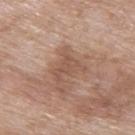This lesion was catalogued during total-body skin photography and was not selected for biopsy. From the upper back. Automated tile analysis of the lesion measured a lesion color around L≈53 a*≈19 b*≈28 in CIELAB, roughly 7 lightness units darker than nearby skin, and a normalized border contrast of about 5. And it measured border irregularity of about 6.5 on a 0–10 scale, internal color variation of about 1.5 on a 0–10 scale, and peripheral color asymmetry of about 0.5. And it measured a classifier nevus-likeness of about 0/100. The patient is a female approximately 75 years of age. A region of skin cropped from a whole-body photographic capture, roughly 15 mm wide. The recorded lesion diameter is about 4 mm. This is a white-light tile.